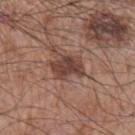Part of a total-body skin-imaging series; this lesion was reviewed on a skin check and was not flagged for biopsy.
On the left upper arm.
About 3.5 mm across.
Imaged with white-light lighting.
The lesion-visualizer software estimated a footprint of about 8 mm², an eccentricity of roughly 0.7, and a shape-asymmetry score of about 0.25 (0 = symmetric). And it measured a lesion color around L≈42 a*≈20 b*≈24 in CIELAB, a lesion–skin lightness drop of about 12, and a normalized border contrast of about 9.
A 15 mm close-up tile from a total-body photography series done for melanoma screening.
A male subject aged 63–67.